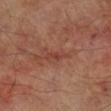<tbp_lesion>
  <biopsy_status>not biopsied; imaged during a skin examination</biopsy_status>
  <lighting>cross-polarized</lighting>
  <lesion_size>
    <long_diameter_mm_approx>2.5</long_diameter_mm_approx>
  </lesion_size>
  <image>
    <source>total-body photography crop</source>
    <field_of_view_mm>15</field_of_view_mm>
  </image>
  <site>right lower leg</site>
  <automated_metrics>
    <eccentricity>0.9</eccentricity>
    <shape_asymmetry>0.35</shape_asymmetry>
    <cielab_L>40</cielab_L>
    <cielab_a>25</cielab_a>
    <cielab_b>26</cielab_b>
    <border_irregularity_0_10>3.5</border_irregularity_0_10>
    <color_variation_0_10>0.0</color_variation_0_10>
    <peripheral_color_asymmetry>0.0</peripheral_color_asymmetry>
    <nevus_likeness_0_100>0</nevus_likeness_0_100>
  </automated_metrics>
  <patient>
    <sex>male</sex>
    <age_approx>70</age_approx>
  </patient>
</tbp_lesion>